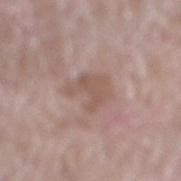{"biopsy_status": "not biopsied; imaged during a skin examination", "image": {"source": "total-body photography crop", "field_of_view_mm": 15}, "lighting": "white-light", "patient": {"sex": "male", "age_approx": 80}, "lesion_size": {"long_diameter_mm_approx": 4.0}, "site": "right lower leg"}